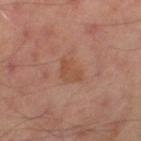A 15 mm crop from a total-body photograph taken for skin-cancer surveillance.
The lesion's longest dimension is about 3.5 mm.
Imaged with cross-polarized lighting.
A male patient approximately 50 years of age.
The lesion is located on the left thigh.